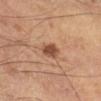Imaged with cross-polarized lighting. About 2.5 mm across. A male subject, aged around 60. On the right lower leg. A 15 mm crop from a total-body photograph taken for skin-cancer surveillance. Automated tile analysis of the lesion measured a lesion area of about 4 mm² and two-axis asymmetry of about 0.25.